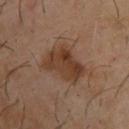Findings:
• acquisition: total-body-photography crop, ~15 mm field of view
• location: the upper back
• subject: male, aged approximately 65
• lighting: cross-polarized illumination
• lesion size: ≈5 mm